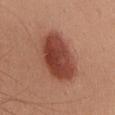Impression:
Recorded during total-body skin imaging; not selected for excision or biopsy.
Acquisition and patient details:
The patient is a male roughly 35 years of age. This is a white-light tile. Cropped from a total-body skin-imaging series; the visible field is about 15 mm. An algorithmic analysis of the crop reported a lesion area of about 21 mm². It also reported an automated nevus-likeness rating near 100 out of 100. The lesion is located on the front of the torso.